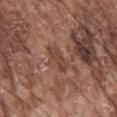Impression: This lesion was catalogued during total-body skin photography and was not selected for biopsy. Image and clinical context: This is a white-light tile. Approximately 3.5 mm at its widest. Cropped from a whole-body photographic skin survey; the tile spans about 15 mm. The lesion is located on the mid back. A male subject, aged 73–77.